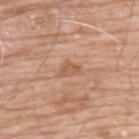follow-up: no biopsy performed (imaged during a skin exam)
site: the upper back
acquisition: 15 mm crop, total-body photography
subject: male, in their mid-60s